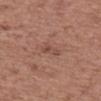<case>
<biopsy_status>not biopsied; imaged during a skin examination</biopsy_status>
</case>